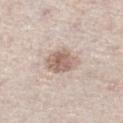Captured during whole-body skin photography for melanoma surveillance; the lesion was not biopsied.
Cropped from a whole-body photographic skin survey; the tile spans about 15 mm.
The lesion is located on the left lower leg.
A male patient aged around 60.